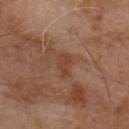workup: no biopsy performed (imaged during a skin exam) | body site: the back | tile lighting: cross-polarized illumination | automated lesion analysis: a footprint of about 4 mm² and an outline eccentricity of about 0.8 (0 = round, 1 = elongated); a lesion color around L≈39 a*≈21 b*≈28 in CIELAB, a lesion–skin lightness drop of about 6, and a normalized border contrast of about 5; a classifier nevus-likeness of about 0/100 | subject: male, roughly 65 years of age | imaging modality: ~15 mm crop, total-body skin-cancer survey.